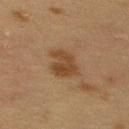<tbp_lesion>
  <biopsy_status>not biopsied; imaged during a skin examination</biopsy_status>
  <lesion_size>
    <long_diameter_mm_approx>4.0</long_diameter_mm_approx>
  </lesion_size>
  <patient>
    <sex>female</sex>
    <age_approx>40</age_approx>
  </patient>
  <automated_metrics>
    <area_mm2_approx>8.5</area_mm2_approx>
    <eccentricity>0.65</eccentricity>
    <shape_asymmetry>0.3</shape_asymmetry>
    <border_irregularity_0_10>3.5</border_irregularity_0_10>
    <color_variation_0_10>3.0</color_variation_0_10>
    <peripheral_color_asymmetry>1.0</peripheral_color_asymmetry>
  </automated_metrics>
  <lighting>cross-polarized</lighting>
  <image>
    <source>total-body photography crop</source>
    <field_of_view_mm>15</field_of_view_mm>
  </image>
  <site>upper back</site>
</tbp_lesion>